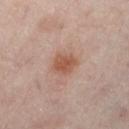<lesion>
  <biopsy_status>not biopsied; imaged during a skin examination</biopsy_status>
  <lighting>cross-polarized</lighting>
  <automated_metrics>
    <border_irregularity_0_10>1.5</border_irregularity_0_10>
  </automated_metrics>
  <lesion_size>
    <long_diameter_mm_approx>3.0</long_diameter_mm_approx>
  </lesion_size>
  <patient>
    <sex>female</sex>
    <age_approx>55</age_approx>
  </patient>
  <site>left lower leg</site>
  <image>
    <source>total-body photography crop</source>
    <field_of_view_mm>15</field_of_view_mm>
  </image>
</lesion>